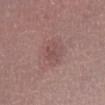Acquisition and patient details: A lesion tile, about 15 mm wide, cut from a 3D total-body photograph. This is a white-light tile. A female patient aged around 40. About 4.5 mm across. On the leg.